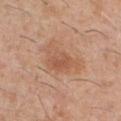Approximately 5 mm at its widest. The tile uses white-light illumination. From the chest. A close-up tile cropped from a whole-body skin photograph, about 15 mm across. A male subject, in their 30s.